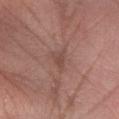Clinical impression:
Imaged during a routine full-body skin examination; the lesion was not biopsied and no histopathology is available.
Acquisition and patient details:
The subject is a male roughly 50 years of age. Imaged with white-light lighting. The lesion is located on the left forearm. This image is a 15 mm lesion crop taken from a total-body photograph. The total-body-photography lesion software estimated a footprint of about 3.5 mm² and an eccentricity of roughly 0.75. The analysis additionally found a border-irregularity index near 4/10 and radial color variation of about 1. The analysis additionally found a nevus-likeness score of about 0/100 and a detector confidence of about 100 out of 100 that the crop contains a lesion.